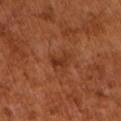<lesion>
<biopsy_status>not biopsied; imaged during a skin examination</biopsy_status>
<lesion_size>
  <long_diameter_mm_approx>2.5</long_diameter_mm_approx>
</lesion_size>
<lighting>cross-polarized</lighting>
<patient>
  <sex>male</sex>
  <age_approx>65</age_approx>
</patient>
<image>
  <source>total-body photography crop</source>
  <field_of_view_mm>15</field_of_view_mm>
</image>
<automated_metrics>
  <eccentricity>0.75</eccentricity>
  <shape_asymmetry>0.45</shape_asymmetry>
  <cielab_L>34</cielab_L>
  <cielab_a>25</cielab_a>
  <cielab_b>32</cielab_b>
  <vs_skin_darker_L>8.0</vs_skin_darker_L>
  <vs_skin_contrast_norm>7.0</vs_skin_contrast_norm>
</automated_metrics>
</lesion>